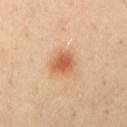follow-up = imaged on a skin check; not biopsied
lighting = cross-polarized
lesion diameter = ~3 mm (longest diameter)
image = 15 mm crop, total-body photography
subject = male, roughly 40 years of age
location = the chest